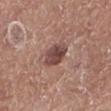Findings:
- notes · total-body-photography surveillance lesion; no biopsy
- tile lighting · white-light
- lesion size · about 3 mm
- site · the left lower leg
- imaging modality · ~15 mm crop, total-body skin-cancer survey
- patient · male, roughly 75 years of age
- image-analysis metrics · an outline eccentricity of about 0.55 (0 = round, 1 = elongated) and two-axis asymmetry of about 0.15; an average lesion color of about L≈45 a*≈21 b*≈23 (CIELAB) and a lesion-to-skin contrast of about 9.5 (normalized; higher = more distinct); a border-irregularity rating of about 1.5/10, internal color variation of about 4 on a 0–10 scale, and radial color variation of about 1.5; a lesion-detection confidence of about 100/100